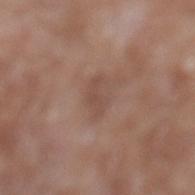Assessment: The lesion was tiled from a total-body skin photograph and was not biopsied. Context: This is a white-light tile. A male patient, aged around 60. The lesion's longest dimension is about 3.5 mm. A 15 mm close-up tile from a total-body photography series done for melanoma screening. Located on the left lower leg.